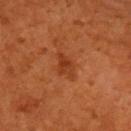A roughly 15 mm field-of-view crop from a total-body skin photograph. The subject is a male about 60 years old. The lesion is located on the upper back. The tile uses cross-polarized illumination. The recorded lesion diameter is about 3 mm.